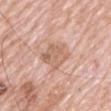Impression:
No biopsy was performed on this lesion — it was imaged during a full skin examination and was not determined to be concerning.
Background:
A region of skin cropped from a whole-body photographic capture, roughly 15 mm wide. Measured at roughly 4.5 mm in maximum diameter. Located on the mid back. The patient is a male in their 80s.